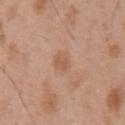Impression:
Recorded during total-body skin imaging; not selected for excision or biopsy.
Acquisition and patient details:
The lesion is located on the chest. A 15 mm close-up tile from a total-body photography series done for melanoma screening. A male patient, in their mid-50s.